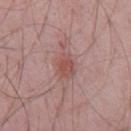Clinical summary:
Measured at roughly 6.5 mm in maximum diameter. The lesion is located on the chest. A male subject roughly 60 years of age. The lesion-visualizer software estimated an average lesion color of about L≈53 a*≈21 b*≈22 (CIELAB) and a lesion-to-skin contrast of about 6 (normalized; higher = more distinct). The analysis additionally found a nevus-likeness score of about 5/100 and lesion-presence confidence of about 100/100. This is a white-light tile. This image is a 15 mm lesion crop taken from a total-body photograph.